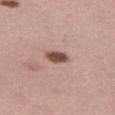Assessment: Recorded during total-body skin imaging; not selected for excision or biopsy. Acquisition and patient details: Approximately 3 mm at its widest. A lesion tile, about 15 mm wide, cut from a 3D total-body photograph. The total-body-photography lesion software estimated a footprint of about 4.5 mm², an eccentricity of roughly 0.75, and a symmetry-axis asymmetry near 0.2. And it measured a lesion color around L≈49 a*≈21 b*≈24 in CIELAB, a lesion–skin lightness drop of about 17, and a normalized border contrast of about 11.5. A female patient, aged 33 to 37. The tile uses white-light illumination. On the leg.